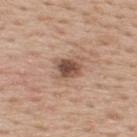biopsy_status: not biopsied; imaged during a skin examination
image:
  source: total-body photography crop
  field_of_view_mm: 15
patient:
  sex: male
  age_approx: 60
site: upper back